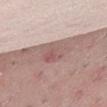Part of a total-body skin-imaging series; this lesion was reviewed on a skin check and was not flagged for biopsy.
The tile uses white-light illumination.
A female subject in their 30s.
Located on the abdomen.
A 15 mm crop from a total-body photograph taken for skin-cancer surveillance.